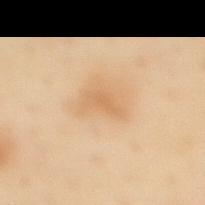follow-up = catalogued during a skin exam; not biopsied
anatomic site = the upper back
acquisition = 15 mm crop, total-body photography
patient = female, in their 60s
size = about 3.5 mm
illumination = cross-polarized illumination